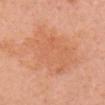Q: Was this lesion biopsied?
A: no biopsy performed (imaged during a skin exam)